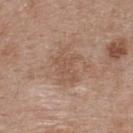<tbp_lesion>
<biopsy_status>not biopsied; imaged during a skin examination</biopsy_status>
</tbp_lesion>